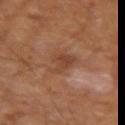notes: imaged on a skin check; not biopsied | site: the left upper arm | subject: male, aged approximately 65 | image: ~15 mm tile from a whole-body skin photo | image-analysis metrics: an outline eccentricity of about 0.85 (0 = round, 1 = elongated) and two-axis asymmetry of about 0.4; an average lesion color of about L≈41 a*≈20 b*≈29 (CIELAB), a lesion–skin lightness drop of about 7, and a lesion-to-skin contrast of about 6 (normalized; higher = more distinct) | tile lighting: cross-polarized | lesion size: ≈4.5 mm.